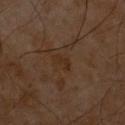A male subject approximately 60 years of age. Cropped from a whole-body photographic skin survey; the tile spans about 15 mm. The lesion is on the front of the torso. The recorded lesion diameter is about 2.5 mm.